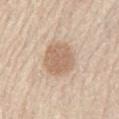biopsy status: total-body-photography surveillance lesion; no biopsy
image: total-body-photography crop, ~15 mm field of view
patient: male, aged around 80
illumination: white-light
anatomic site: the back
lesion size: about 4 mm